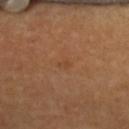Q: Is there a histopathology result?
A: no biopsy performed (imaged during a skin exam)